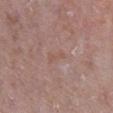The lesion was tiled from a total-body skin photograph and was not biopsied. A 15 mm close-up extracted from a 3D total-body photography capture. About 2.5 mm across. From the right lower leg. A male subject approximately 65 years of age.